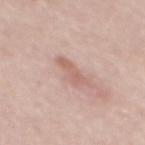This lesion was catalogued during total-body skin photography and was not selected for biopsy. The lesion is on the back. The lesion's longest dimension is about 4.5 mm. The patient is a female aged 38–42. This image is a 15 mm lesion crop taken from a total-body photograph. Captured under white-light illumination.